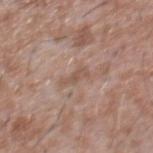Case summary:
• biopsy status: imaged on a skin check; not biopsied
• image-analysis metrics: an outline eccentricity of about 0.9 (0 = round, 1 = elongated); a lesion color around L≈53 a*≈18 b*≈26 in CIELAB, roughly 7 lightness units darker than nearby skin, and a normalized border contrast of about 6; a color-variation rating of about 0/10 and radial color variation of about 0
• image source: ~15 mm crop, total-body skin-cancer survey
• tile lighting: white-light illumination
• site: the chest
• patient: male, aged around 45
• size: about 3 mm